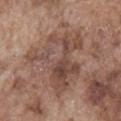Notes:
– automated lesion analysis: about 9 CIELAB-L* units darker than the surrounding skin and a normalized border contrast of about 7; a classifier nevus-likeness of about 0/100 and lesion-presence confidence of about 85/100
– imaging modality: total-body-photography crop, ~15 mm field of view
– site: the front of the torso
– subject: male, roughly 75 years of age